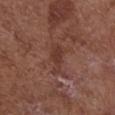notes=no biopsy performed (imaged during a skin exam); subject=female, about 80 years old; body site=the chest; acquisition=~15 mm crop, total-body skin-cancer survey; tile lighting=white-light illumination.